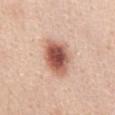follow-up: total-body-photography surveillance lesion; no biopsy
imaging modality: 15 mm crop, total-body photography
patient: female, aged approximately 45
tile lighting: white-light
location: the chest
lesion diameter: ≈5.5 mm